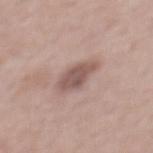Case summary:
• workup — no biopsy performed (imaged during a skin exam)
• patient — male, aged 58–62
• automated lesion analysis — a lesion area of about 8 mm² and an outline eccentricity of about 0.85 (0 = round, 1 = elongated); an average lesion color of about L≈54 a*≈18 b*≈22 (CIELAB) and roughly 11 lightness units darker than nearby skin; an automated nevus-likeness rating near 10 out of 100
• body site — the mid back
• size — ≈4.5 mm
• image — ~15 mm crop, total-body skin-cancer survey